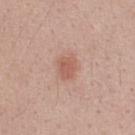Q: Was this lesion biopsied?
A: catalogued during a skin exam; not biopsied
Q: Where on the body is the lesion?
A: the upper back
Q: What are the patient's age and sex?
A: male, aged 38 to 42
Q: What is the imaging modality?
A: ~15 mm crop, total-body skin-cancer survey
Q: Automated lesion metrics?
A: a footprint of about 5 mm², an outline eccentricity of about 0.55 (0 = round, 1 = elongated), and two-axis asymmetry of about 0.3; a mean CIELAB color near L≈58 a*≈24 b*≈28, about 9 CIELAB-L* units darker than the surrounding skin, and a normalized border contrast of about 6.5; a nevus-likeness score of about 80/100 and lesion-presence confidence of about 100/100